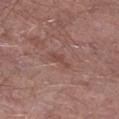Case summary:
• notes · no biopsy performed (imaged during a skin exam)
• tile lighting · white-light
• subject · male, aged 68 to 72
• location · the leg
• image source · total-body-photography crop, ~15 mm field of view